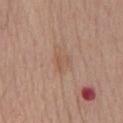follow-up = catalogued during a skin exam; not biopsied
subject = male, roughly 70 years of age
lesion size = about 2.5 mm
location = the chest
imaging modality = ~15 mm tile from a whole-body skin photo